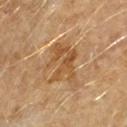tile lighting: cross-polarized illumination
image: ~15 mm tile from a whole-body skin photo
TBP lesion metrics: a lesion area of about 13 mm², an outline eccentricity of about 0.75 (0 = round, 1 = elongated), and two-axis asymmetry of about 0.4; a nevus-likeness score of about 0/100 and lesion-presence confidence of about 75/100
diameter: ~5.5 mm (longest diameter)
location: the left forearm
subject: female, aged 58–62